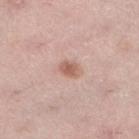Imaged during a routine full-body skin examination; the lesion was not biopsied and no histopathology is available.
The lesion is on the left lower leg.
The subject is a female aged around 50.
A roughly 15 mm field-of-view crop from a total-body skin photograph.